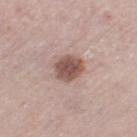Impression:
Recorded during total-body skin imaging; not selected for excision or biopsy.